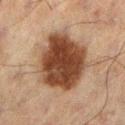Clinical impression:
The lesion was photographed on a routine skin check and not biopsied; there is no pathology result.
Background:
Automated tile analysis of the lesion measured a lesion area of about 36 mm², a shape eccentricity near 0.5, and two-axis asymmetry of about 0.15. The analysis additionally found an average lesion color of about L≈33 a*≈16 b*≈25 (CIELAB) and roughly 14 lightness units darker than nearby skin. The software also gave a classifier nevus-likeness of about 95/100 and lesion-presence confidence of about 100/100. This image is a 15 mm lesion crop taken from a total-body photograph. Longest diameter approximately 7 mm. From the leg. A male subject aged around 60. Captured under cross-polarized illumination.